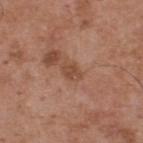follow-up: no biopsy performed (imaged during a skin exam)
subject: male, in their mid-50s
image: ~15 mm crop, total-body skin-cancer survey
diameter: about 2.5 mm
automated metrics: an area of roughly 3.5 mm², an outline eccentricity of about 0.75 (0 = round, 1 = elongated), and a shape-asymmetry score of about 0.2 (0 = symmetric); roughly 8 lightness units darker than nearby skin and a normalized lesion–skin contrast near 6.5; a nevus-likeness score of about 0/100 and lesion-presence confidence of about 100/100
anatomic site: the back
tile lighting: white-light illumination